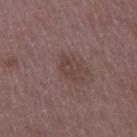biopsy_status: not biopsied; imaged during a skin examination
lighting: white-light
patient:
  sex: female
  age_approx: 45
image:
  source: total-body photography crop
  field_of_view_mm: 15
site: right upper arm